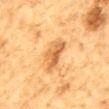A lesion tile, about 15 mm wide, cut from a 3D total-body photograph. Captured under cross-polarized illumination. About 6 mm across. A male subject aged approximately 85 or older. Located on the mid back.